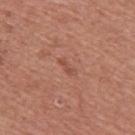– workup · no biopsy performed (imaged during a skin exam)
– imaging modality · total-body-photography crop, ~15 mm field of view
– location · the front of the torso
– subject · male, in their mid-40s